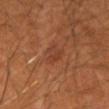biopsy status — total-body-photography surveillance lesion; no biopsy | imaging modality — total-body-photography crop, ~15 mm field of view | body site — the left forearm | patient — male, aged approximately 50 | automated lesion analysis — a lesion area of about 4.5 mm², an outline eccentricity of about 0.85 (0 = round, 1 = elongated), and a symmetry-axis asymmetry near 0.35; a border-irregularity rating of about 4/10, internal color variation of about 3 on a 0–10 scale, and radial color variation of about 1; an automated nevus-likeness rating near 0 out of 100 and lesion-presence confidence of about 95/100 | lesion diameter — ~3.5 mm (longest diameter).